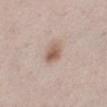This lesion was catalogued during total-body skin photography and was not selected for biopsy. A roughly 15 mm field-of-view crop from a total-body skin photograph. The tile uses white-light illumination. A female patient, aged around 40. Located on the left lower leg. Longest diameter approximately 3 mm.